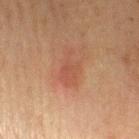Q: Was a biopsy performed?
A: no biopsy performed (imaged during a skin exam)